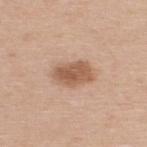body site=the upper back
diameter=≈4 mm
acquisition=total-body-photography crop, ~15 mm field of view
lighting=white-light
subject=male, in their mid- to late 40s